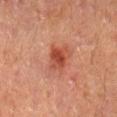<lesion>
  <biopsy_status>not biopsied; imaged during a skin examination</biopsy_status>
  <image>
    <source>total-body photography crop</source>
    <field_of_view_mm>15</field_of_view_mm>
  </image>
  <patient>
    <sex>male</sex>
    <age_approx>60</age_approx>
  </patient>
  <automated_metrics>
    <nevus_likeness_0_100>80</nevus_likeness_0_100>
    <lesion_detection_confidence_0_100>100</lesion_detection_confidence_0_100>
  </automated_metrics>
  <site>mid back</site>
  <lighting>cross-polarized</lighting>
  <lesion_size>
    <long_diameter_mm_approx>3.0</long_diameter_mm_approx>
  </lesion_size>
</lesion>